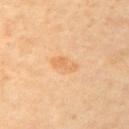Notes:
* follow-up · no biopsy performed (imaged during a skin exam)
* imaging modality · 15 mm crop, total-body photography
* patient · female, aged 58–62
* TBP lesion metrics · an area of roughly 4 mm², an outline eccentricity of about 0.85 (0 = round, 1 = elongated), and a shape-asymmetry score of about 0.3 (0 = symmetric); a classifier nevus-likeness of about 25/100 and a detector confidence of about 100 out of 100 that the crop contains a lesion
* tile lighting · cross-polarized
* size · ~3 mm (longest diameter)
* body site · the left upper arm